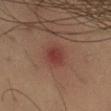Q: Is there a histopathology result?
A: total-body-photography surveillance lesion; no biopsy
Q: What is the anatomic site?
A: the leg
Q: Lesion size?
A: ~2.5 mm (longest diameter)
Q: Illumination type?
A: cross-polarized
Q: Automated lesion metrics?
A: a lesion color around L≈33 a*≈24 b*≈22 in CIELAB, a lesion–skin lightness drop of about 7, and a normalized border contrast of about 7; a border-irregularity rating of about 1.5/10, a within-lesion color-variation index near 1.5/10, and peripheral color asymmetry of about 0.5; a classifier nevus-likeness of about 5/100 and a lesion-detection confidence of about 100/100
Q: Patient demographics?
A: male, approximately 35 years of age
Q: What is the imaging modality?
A: 15 mm crop, total-body photography